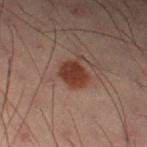Imaged during a routine full-body skin examination; the lesion was not biopsied and no histopathology is available. A lesion tile, about 15 mm wide, cut from a 3D total-body photograph. Located on the left thigh. About 3 mm across. An algorithmic analysis of the crop reported an average lesion color of about L≈29 a*≈19 b*≈22 (CIELAB). And it measured a border-irregularity index near 1.5/10, internal color variation of about 2.5 on a 0–10 scale, and radial color variation of about 1. The analysis additionally found a nevus-likeness score of about 95/100 and a lesion-detection confidence of about 100/100. Captured under cross-polarized illumination. The subject is a male approximately 55 years of age.